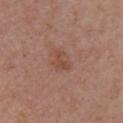Clinical impression:
Recorded during total-body skin imaging; not selected for excision or biopsy.
Background:
A female subject aged around 55. Located on the chest. Measured at roughly 2.5 mm in maximum diameter. Captured under white-light illumination. A 15 mm crop from a total-body photograph taken for skin-cancer surveillance.